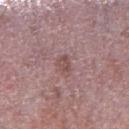| field | value |
|---|---|
| biopsy status | total-body-photography surveillance lesion; no biopsy |
| imaging modality | 15 mm crop, total-body photography |
| anatomic site | the leg |
| lesion size | ≈2.5 mm |
| subject | male, in their mid- to late 60s |